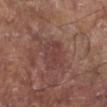Case summary:
* notes · total-body-photography surveillance lesion; no biopsy
* location · the left lower leg
* subject · male, aged 68–72
* acquisition · ~15 mm crop, total-body skin-cancer survey